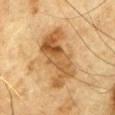Case summary:
* notes — catalogued during a skin exam; not biopsied
* lesion size — ≈7.5 mm
* site — the chest
* subject — male, aged 83 or over
* acquisition — ~15 mm tile from a whole-body skin photo